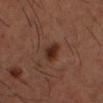{"biopsy_status": "not biopsied; imaged during a skin examination", "image": {"source": "total-body photography crop", "field_of_view_mm": 15}, "site": "head or neck", "automated_metrics": {"area_mm2_approx": 5.0, "eccentricity": 0.65, "border_irregularity_0_10": 2.0, "color_variation_0_10": 4.0}, "patient": {"sex": "male", "age_approx": 35}, "lesion_size": {"long_diameter_mm_approx": 3.0}}